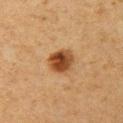biopsy status: catalogued during a skin exam; not biopsied
site: the left upper arm
acquisition: ~15 mm crop, total-body skin-cancer survey
subject: female, aged around 40
diameter: ≈3.5 mm
illumination: cross-polarized